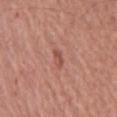Clinical impression:
No biopsy was performed on this lesion — it was imaged during a full skin examination and was not determined to be concerning.
Context:
Cropped from a total-body skin-imaging series; the visible field is about 15 mm. The lesion is on the abdomen. A male subject, aged approximately 65.